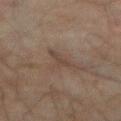{
  "biopsy_status": "not biopsied; imaged during a skin examination",
  "lesion_size": {
    "long_diameter_mm_approx": 4.0
  },
  "patient": {
    "sex": "male",
    "age_approx": 75
  },
  "automated_metrics": {
    "area_mm2_approx": 4.0,
    "shape_asymmetry": 0.55,
    "cielab_L": 34,
    "cielab_a": 12,
    "cielab_b": 20,
    "vs_skin_darker_L": 5.0,
    "vs_skin_contrast_norm": 5.5
  },
  "site": "abdomen",
  "lighting": "cross-polarized",
  "image": {
    "source": "total-body photography crop",
    "field_of_view_mm": 15
  }
}